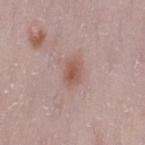A female subject, aged approximately 30. On the left thigh. A 15 mm close-up extracted from a 3D total-body photography capture.